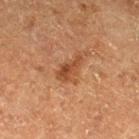– workup — total-body-photography surveillance lesion; no biopsy
– illumination — cross-polarized
– location — the right thigh
– patient — male, aged around 75
– image source — ~15 mm crop, total-body skin-cancer survey
– TBP lesion metrics — a lesion area of about 5.5 mm², an outline eccentricity of about 0.9 (0 = round, 1 = elongated), and a shape-asymmetry score of about 0.4 (0 = symmetric); a mean CIELAB color near L≈38 a*≈21 b*≈30, roughly 8 lightness units darker than nearby skin, and a lesion-to-skin contrast of about 7 (normalized; higher = more distinct); border irregularity of about 4.5 on a 0–10 scale, internal color variation of about 2.5 on a 0–10 scale, and peripheral color asymmetry of about 1; a nevus-likeness score of about 70/100
– lesion diameter — ~4 mm (longest diameter)